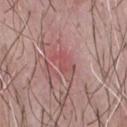| field | value |
|---|---|
| subject | male, in their 50s |
| image | 15 mm crop, total-body photography |
| automated metrics | an outline eccentricity of about 0.85 (0 = round, 1 = elongated) and a shape-asymmetry score of about 0.45 (0 = symmetric); a nevus-likeness score of about 0/100 |
| location | the chest |